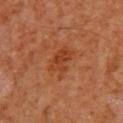Notes:
– notes — catalogued during a skin exam; not biopsied
– image source — ~15 mm crop, total-body skin-cancer survey
– body site — the upper back
– patient — male, in their 60s
– illumination — cross-polarized illumination